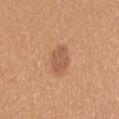notes: imaged on a skin check; not biopsied
lesion size: ~3.5 mm (longest diameter)
image: ~15 mm crop, total-body skin-cancer survey
image-analysis metrics: a classifier nevus-likeness of about 40/100 and lesion-presence confidence of about 100/100
subject: female, in their mid- to late 30s
illumination: white-light
body site: the right upper arm